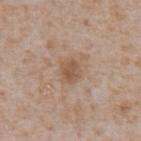Q: Is there a histopathology result?
A: total-body-photography surveillance lesion; no biopsy
Q: Patient demographics?
A: male, approximately 65 years of age
Q: Automated lesion metrics?
A: a footprint of about 5 mm², an outline eccentricity of about 0.6 (0 = round, 1 = elongated), and a symmetry-axis asymmetry near 0.2; a border-irregularity index near 1.5/10 and a peripheral color-asymmetry measure near 1
Q: What kind of image is this?
A: total-body-photography crop, ~15 mm field of view
Q: How large is the lesion?
A: ≈2.5 mm
Q: Lesion location?
A: the abdomen
Q: What lighting was used for the tile?
A: white-light illumination